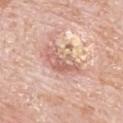Part of a total-body skin-imaging series; this lesion was reviewed on a skin check and was not flagged for biopsy. A 15 mm close-up extracted from a 3D total-body photography capture. A male subject aged 78 to 82. From the mid back. Longest diameter approximately 5 mm. The tile uses white-light illumination.